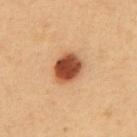Q: Automated lesion metrics?
A: an area of roughly 9 mm², an eccentricity of roughly 0.5, and a symmetry-axis asymmetry near 0.15; a border-irregularity index near 1/10, a within-lesion color-variation index near 4.5/10, and a peripheral color-asymmetry measure near 1
Q: How was this image acquired?
A: total-body-photography crop, ~15 mm field of view
Q: Patient demographics?
A: male, in their 50s
Q: What is the anatomic site?
A: the abdomen
Q: Illumination type?
A: cross-polarized illumination
Q: Lesion size?
A: ~3.5 mm (longest diameter)